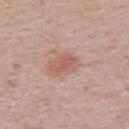The lesion was photographed on a routine skin check and not biopsied; there is no pathology result. A 15 mm close-up tile from a total-body photography series done for melanoma screening. Imaged with white-light lighting. A male subject aged around 40. The total-body-photography lesion software estimated an area of roughly 7 mm², an outline eccentricity of about 0.65 (0 = round, 1 = elongated), and a symmetry-axis asymmetry near 0.2. The analysis additionally found a mean CIELAB color near L≈58 a*≈22 b*≈26 and roughly 8 lightness units darker than nearby skin. It also reported a border-irregularity rating of about 2/10, a color-variation rating of about 4/10, and radial color variation of about 1.5. And it measured a classifier nevus-likeness of about 65/100 and a lesion-detection confidence of about 100/100. From the upper back. The recorded lesion diameter is about 3.5 mm.